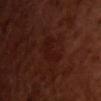Clinical summary: This image is a 15 mm lesion crop taken from a total-body photograph. Automated tile analysis of the lesion measured an average lesion color of about L≈17 a*≈22 b*≈21 (CIELAB) and about 4 CIELAB-L* units darker than the surrounding skin. The analysis additionally found a border-irregularity rating of about 5/10, a within-lesion color-variation index near 2/10, and radial color variation of about 1. This is a cross-polarized tile. The lesion is located on the chest. A female subject aged approximately 55.